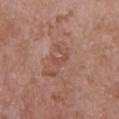Q: Was a biopsy performed?
A: catalogued during a skin exam; not biopsied
Q: What is the imaging modality?
A: ~15 mm tile from a whole-body skin photo
Q: How was the tile lit?
A: white-light
Q: Patient demographics?
A: female, roughly 70 years of age
Q: What is the lesion's diameter?
A: ≈2.5 mm
Q: Where on the body is the lesion?
A: the right upper arm